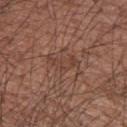| feature | finding |
|---|---|
| follow-up | total-body-photography surveillance lesion; no biopsy |
| subject | male, aged 63–67 |
| imaging modality | ~15 mm tile from a whole-body skin photo |
| TBP lesion metrics | an average lesion color of about L≈42 a*≈20 b*≈26 (CIELAB) and a lesion-to-skin contrast of about 5 (normalized; higher = more distinct); a border-irregularity index near 5.5/10 and peripheral color asymmetry of about 0.5; a classifier nevus-likeness of about 0/100 and a lesion-detection confidence of about 70/100 |
| lighting | white-light illumination |
| location | the left upper arm |
| lesion diameter | about 4 mm |